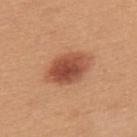Assessment: Captured during whole-body skin photography for melanoma surveillance; the lesion was not biopsied. Clinical summary: A female patient aged approximately 30. This is a white-light tile. A 15 mm crop from a total-body photograph taken for skin-cancer surveillance. On the upper back. Automated image analysis of the tile measured an outline eccentricity of about 0.75 (0 = round, 1 = elongated) and two-axis asymmetry of about 0.1. The analysis additionally found peripheral color asymmetry of about 2. The lesion's longest dimension is about 5.5 mm.